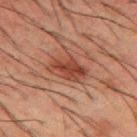follow-up = no biopsy performed (imaged during a skin exam)
location = the mid back
subject = male, roughly 50 years of age
image = ~15 mm crop, total-body skin-cancer survey